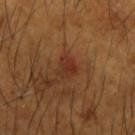follow-up: imaged on a skin check; not biopsied
image source: ~15 mm crop, total-body skin-cancer survey
subject: male, aged around 65
location: the left forearm